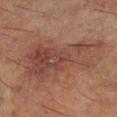Case summary:
- follow-up · imaged on a skin check; not biopsied
- imaging modality · ~15 mm tile from a whole-body skin photo
- lesion size · ≈10.5 mm
- patient · male, about 60 years old
- location · the right lower leg